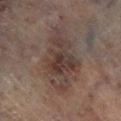Impression:
Part of a total-body skin-imaging series; this lesion was reviewed on a skin check and was not flagged for biopsy.
Image and clinical context:
A male subject, aged 63 to 67. The recorded lesion diameter is about 8.5 mm. The lesion is on the right lower leg. A 15 mm close-up extracted from a 3D total-body photography capture.